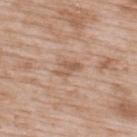This is a white-light tile.
Approximately 3 mm at its widest.
Cropped from a whole-body photographic skin survey; the tile spans about 15 mm.
On the back.
A male subject aged 48 to 52.